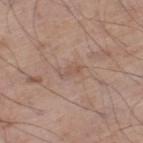site: left thigh
image:
  source: total-body photography crop
  field_of_view_mm: 15
lesion_size:
  long_diameter_mm_approx: 2.5
patient:
  sex: male
  age_approx: 70